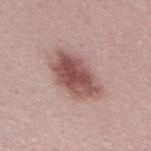A male subject, in their 30s. A 15 mm close-up extracted from a 3D total-body photography capture. Automated tile analysis of the lesion measured a footprint of about 17 mm² and a shape eccentricity near 0.8. The software also gave radial color variation of about 2.